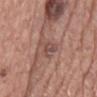<record>
  <biopsy_status>not biopsied; imaged during a skin examination</biopsy_status>
  <lighting>white-light</lighting>
  <site>back</site>
  <lesion_size>
    <long_diameter_mm_approx>3.5</long_diameter_mm_approx>
  </lesion_size>
  <patient>
    <sex>male</sex>
    <age_approx>75</age_approx>
  </patient>
  <image>
    <source>total-body photography crop</source>
    <field_of_view_mm>15</field_of_view_mm>
  </image>
</record>